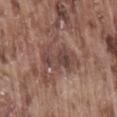<case>
<lesion_size>
  <long_diameter_mm_approx>5.0</long_diameter_mm_approx>
</lesion_size>
<patient>
  <sex>male</sex>
  <age_approx>75</age_approx>
</patient>
<automated_metrics>
  <eccentricity>0.85</eccentricity>
  <shape_asymmetry>0.35</shape_asymmetry>
  <cielab_L>43</cielab_L>
  <cielab_a>18</cielab_a>
  <cielab_b>22</cielab_b>
  <vs_skin_contrast_norm>7.0</vs_skin_contrast_norm>
  <peripheral_color_asymmetry>2.0</peripheral_color_asymmetry>
</automated_metrics>
<lighting>white-light</lighting>
<image>
  <source>total-body photography crop</source>
  <field_of_view_mm>15</field_of_view_mm>
</image>
<site>lower back</site>
</case>